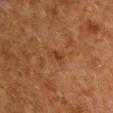Q: Was this lesion biopsied?
A: no biopsy performed (imaged during a skin exam)
Q: Lesion location?
A: the left upper arm
Q: What is the lesion's diameter?
A: ≈2.5 mm
Q: How was the tile lit?
A: cross-polarized illumination
Q: How was this image acquired?
A: total-body-photography crop, ~15 mm field of view
Q: Patient demographics?
A: male, aged around 60
Q: What did automated image analysis measure?
A: two-axis asymmetry of about 0.3; border irregularity of about 3 on a 0–10 scale, internal color variation of about 2.5 on a 0–10 scale, and radial color variation of about 1